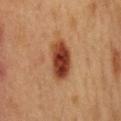Case summary:
* lighting: cross-polarized
* anatomic site: the chest
* imaging modality: ~15 mm tile from a whole-body skin photo
* size: about 5 mm
* patient: female, about 40 years old
* automated lesion analysis: an area of roughly 11 mm², a shape eccentricity near 0.85, and a symmetry-axis asymmetry near 0.2